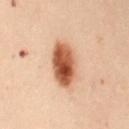image: 15 mm crop, total-body photography | patient: female, about 50 years old | diameter: about 5.5 mm | body site: the mid back | illumination: cross-polarized.